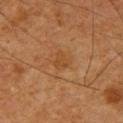Case summary:
* workup: total-body-photography surveillance lesion; no biopsy
* automated lesion analysis: two-axis asymmetry of about 0.4; a lesion color around L≈42 a*≈20 b*≈35 in CIELAB, a lesion–skin lightness drop of about 5, and a normalized lesion–skin contrast near 5; a nevus-likeness score of about 0/100 and lesion-presence confidence of about 100/100
* image source: ~15 mm tile from a whole-body skin photo
* body site: the left upper arm
* patient: male, about 60 years old